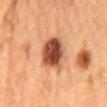{"biopsy_status": "not biopsied; imaged during a skin examination", "lesion_size": {"long_diameter_mm_approx": 5.5}, "site": "mid back", "image": {"source": "total-body photography crop", "field_of_view_mm": 15}, "automated_metrics": {"vs_skin_darker_L": 17.0, "vs_skin_contrast_norm": 12.0, "border_irregularity_0_10": 2.0, "color_variation_0_10": 8.0, "peripheral_color_asymmetry": 2.5, "nevus_likeness_0_100": 50}, "patient": {"sex": "female", "age_approx": 60}}